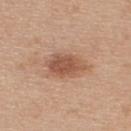Q: Is there a histopathology result?
A: catalogued during a skin exam; not biopsied
Q: Who is the patient?
A: female, aged 38 to 42
Q: What is the anatomic site?
A: the upper back
Q: What is the imaging modality?
A: ~15 mm crop, total-body skin-cancer survey